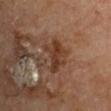Notes:
– follow-up — total-body-photography surveillance lesion; no biopsy
– anatomic site — the right upper arm
– illumination — cross-polarized
– acquisition — total-body-photography crop, ~15 mm field of view
– lesion size — ≈4.5 mm
– subject — male, aged 68 to 72